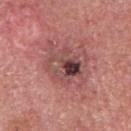Clinical impression: No biopsy was performed on this lesion — it was imaged during a full skin examination and was not determined to be concerning. Context: A region of skin cropped from a whole-body photographic capture, roughly 15 mm wide. The lesion is on the head or neck. A male subject, approximately 60 years of age.